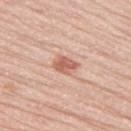Clinical impression: Part of a total-body skin-imaging series; this lesion was reviewed on a skin check and was not flagged for biopsy. Background: On the left thigh. This is a white-light tile. The lesion-visualizer software estimated about 12 CIELAB-L* units darker than the surrounding skin. The software also gave a classifier nevus-likeness of about 80/100 and a lesion-detection confidence of about 100/100. Longest diameter approximately 3 mm. A lesion tile, about 15 mm wide, cut from a 3D total-body photograph. A male patient aged 68–72.